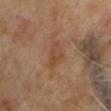Impression: The lesion was photographed on a routine skin check and not biopsied; there is no pathology result. Image and clinical context: The lesion-visualizer software estimated a footprint of about 5.5 mm², a shape eccentricity near 0.85, and a symmetry-axis asymmetry near 0.35. It also reported a border-irregularity index near 4/10 and radial color variation of about 1.5. The lesion's longest dimension is about 4 mm. A male patient in their mid-60s. The lesion is on the arm. A 15 mm crop from a total-body photograph taken for skin-cancer surveillance.